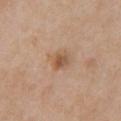The lesion was photographed on a routine skin check and not biopsied; there is no pathology result.
A female patient, aged 38–42.
On the chest.
An algorithmic analysis of the crop reported a lesion area of about 6.5 mm² and an outline eccentricity of about 0.7 (0 = round, 1 = elongated). The analysis additionally found border irregularity of about 2 on a 0–10 scale, internal color variation of about 5 on a 0–10 scale, and peripheral color asymmetry of about 1.5. The software also gave a nevus-likeness score of about 65/100 and a detector confidence of about 100 out of 100 that the crop contains a lesion.
A lesion tile, about 15 mm wide, cut from a 3D total-body photograph.
This is a white-light tile.